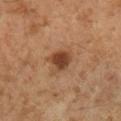Assessment:
No biopsy was performed on this lesion — it was imaged during a full skin examination and was not determined to be concerning.
Context:
This is a cross-polarized tile. Longest diameter approximately 3.5 mm. The subject is a male roughly 60 years of age. The total-body-photography lesion software estimated an eccentricity of roughly 0.55. It also reported an average lesion color of about L≈39 a*≈20 b*≈30 (CIELAB), about 12 CIELAB-L* units darker than the surrounding skin, and a normalized border contrast of about 10. And it measured a nevus-likeness score of about 95/100 and a detector confidence of about 100 out of 100 that the crop contains a lesion. A 15 mm close-up extracted from a 3D total-body photography capture. The lesion is located on the right lower leg.